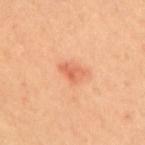Impression: No biopsy was performed on this lesion — it was imaged during a full skin examination and was not determined to be concerning. Image and clinical context: About 3 mm across. The total-body-photography lesion software estimated a lesion area of about 4.5 mm² and a shape-asymmetry score of about 0.4 (0 = symmetric). It also reported a nevus-likeness score of about 35/100. Located on the upper back. This image is a 15 mm lesion crop taken from a total-body photograph. The subject is a female aged approximately 40. This is a cross-polarized tile.